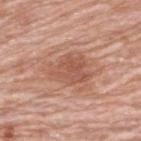Assessment: The lesion was tiled from a total-body skin photograph and was not biopsied. Context: A male subject aged approximately 80. This image is a 15 mm lesion crop taken from a total-body photograph. Located on the upper back.